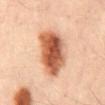Findings:
- notes — total-body-photography surveillance lesion; no biopsy
- subject — male, aged 48 to 52
- body site — the mid back
- diameter — about 7 mm
- illumination — cross-polarized illumination
- imaging modality — ~15 mm crop, total-body skin-cancer survey